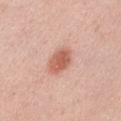The lesion was photographed on a routine skin check and not biopsied; there is no pathology result. The patient is a male aged 38 to 42. About 4 mm across. Imaged with white-light lighting. A 15 mm close-up extracted from a 3D total-body photography capture. On the arm.